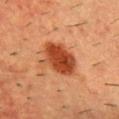This is a cross-polarized tile.
A 15 mm close-up tile from a total-body photography series done for melanoma screening.
The lesion is on the back.
A male subject, in their mid-50s.
Longest diameter approximately 5 mm.